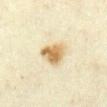Captured during whole-body skin photography for melanoma surveillance; the lesion was not biopsied. The tile uses cross-polarized illumination. A female patient, aged 33 to 37. A 15 mm crop from a total-body photograph taken for skin-cancer surveillance. Located on the front of the torso.